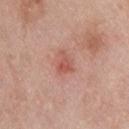Q: Was a biopsy performed?
A: total-body-photography surveillance lesion; no biopsy
Q: Lesion size?
A: ≈3 mm
Q: What are the patient's age and sex?
A: male, approximately 45 years of age
Q: Where on the body is the lesion?
A: the upper back
Q: How was the tile lit?
A: white-light illumination
Q: What is the imaging modality?
A: total-body-photography crop, ~15 mm field of view